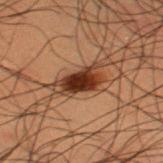Part of a total-body skin-imaging series; this lesion was reviewed on a skin check and was not flagged for biopsy. The patient is a male aged approximately 50. A lesion tile, about 15 mm wide, cut from a 3D total-body photograph. The lesion is located on the left thigh. Imaged with cross-polarized lighting. The lesion-visualizer software estimated a mean CIELAB color near L≈25 a*≈20 b*≈25. And it measured a border-irregularity rating of about 2.5/10, internal color variation of about 4.5 on a 0–10 scale, and radial color variation of about 1.5. The software also gave a classifier nevus-likeness of about 100/100.